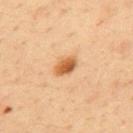This lesion was catalogued during total-body skin photography and was not selected for biopsy. Located on the mid back. About 3 mm across. Cropped from a whole-body photographic skin survey; the tile spans about 15 mm. This is a cross-polarized tile. An algorithmic analysis of the crop reported a lesion–skin lightness drop of about 13. The analysis additionally found a border-irregularity rating of about 2.5/10, a color-variation rating of about 4/10, and peripheral color asymmetry of about 1.5. A male subject, roughly 35 years of age.